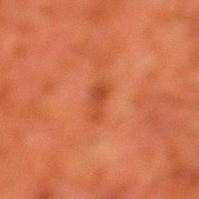Q: Is there a histopathology result?
A: catalogued during a skin exam; not biopsied
Q: How was this image acquired?
A: total-body-photography crop, ~15 mm field of view
Q: What did automated image analysis measure?
A: border irregularity of about 5.5 on a 0–10 scale and peripheral color asymmetry of about 0.5; an automated nevus-likeness rating near 0 out of 100 and a lesion-detection confidence of about 100/100
Q: Where on the body is the lesion?
A: the left lower leg
Q: Patient demographics?
A: male, aged 78–82
Q: Illumination type?
A: cross-polarized illumination
Q: How large is the lesion?
A: about 2.5 mm